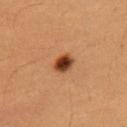The lesion was photographed on a routine skin check and not biopsied; there is no pathology result. On the arm. Captured under cross-polarized illumination. A 15 mm crop from a total-body photograph taken for skin-cancer surveillance. Approximately 2.5 mm at its widest. The lesion-visualizer software estimated an area of roughly 4 mm², an outline eccentricity of about 0.65 (0 = round, 1 = elongated), and two-axis asymmetry of about 0.15. It also reported roughly 19 lightness units darker than nearby skin and a lesion-to-skin contrast of about 13.5 (normalized; higher = more distinct). The software also gave a border-irregularity index near 1.5/10 and peripheral color asymmetry of about 1.5. The subject is a female aged around 40.